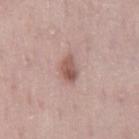<record>
<biopsy_status>not biopsied; imaged during a skin examination</biopsy_status>
</record>